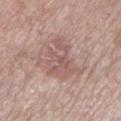Part of a total-body skin-imaging series; this lesion was reviewed on a skin check and was not flagged for biopsy.
Approximately 5 mm at its widest.
On the leg.
A 15 mm close-up tile from a total-body photography series done for melanoma screening.
This is a white-light tile.
A female patient, aged 63 to 67.
The lesion-visualizer software estimated an area of roughly 12 mm², an eccentricity of roughly 0.75, and a symmetry-axis asymmetry near 0.45. The software also gave a lesion color around L≈56 a*≈19 b*≈22 in CIELAB, roughly 8 lightness units darker than nearby skin, and a lesion-to-skin contrast of about 5.5 (normalized; higher = more distinct). And it measured lesion-presence confidence of about 95/100.